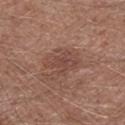No biopsy was performed on this lesion — it was imaged during a full skin examination and was not determined to be concerning. A 15 mm close-up tile from a total-body photography series done for melanoma screening. Measured at roughly 4 mm in maximum diameter. The subject is a male approximately 60 years of age. Located on the right lower leg. Imaged with white-light lighting.